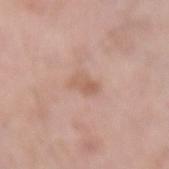imaging modality = 15 mm crop, total-body photography
size = ~3 mm (longest diameter)
subject = male, about 60 years old
lighting = white-light illumination
image-analysis metrics = a footprint of about 4.5 mm²; a border-irregularity index near 3/10, internal color variation of about 3 on a 0–10 scale, and a peripheral color-asymmetry measure near 1; a classifier nevus-likeness of about 0/100 and a detector confidence of about 100 out of 100 that the crop contains a lesion
body site = the left forearm